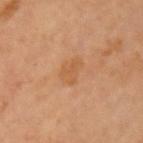| key | value |
|---|---|
| biopsy status | catalogued during a skin exam; not biopsied |
| location | the arm |
| illumination | cross-polarized illumination |
| imaging modality | ~15 mm crop, total-body skin-cancer survey |
| patient | female, aged around 70 |